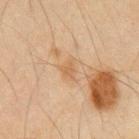This lesion was catalogued during total-body skin photography and was not selected for biopsy.
A male patient, in their mid- to late 60s.
Longest diameter approximately 2.5 mm.
The tile uses cross-polarized illumination.
From the front of the torso.
A lesion tile, about 15 mm wide, cut from a 3D total-body photograph.